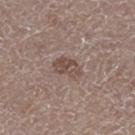Notes:
- biopsy status: no biopsy performed (imaged during a skin exam)
- size: ~3.5 mm (longest diameter)
- body site: the left thigh
- lighting: white-light
- acquisition: ~15 mm crop, total-body skin-cancer survey
- automated metrics: a border-irregularity index near 3/10, internal color variation of about 4 on a 0–10 scale, and peripheral color asymmetry of about 1.5; a classifier nevus-likeness of about 5/100
- subject: male, about 75 years old